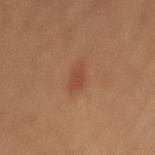biopsy_status: not biopsied; imaged during a skin examination
site: abdomen
image:
  source: total-body photography crop
  field_of_view_mm: 15
patient:
  sex: male
  age_approx: 60